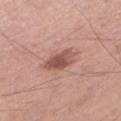notes = imaged on a skin check; not biopsied | site = the left lower leg | TBP lesion metrics = a border-irregularity rating of about 2.5/10, a color-variation rating of about 4.5/10, and radial color variation of about 1.5; a nevus-likeness score of about 70/100 and a lesion-detection confidence of about 100/100 | illumination = white-light illumination | imaging modality = ~15 mm crop, total-body skin-cancer survey | subject = male, in their 50s | diameter = ≈4.5 mm.